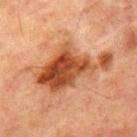Impression:
Recorded during total-body skin imaging; not selected for excision or biopsy.
Image and clinical context:
This is a cross-polarized tile. A male subject approximately 65 years of age. Measured at roughly 9.5 mm in maximum diameter. From the front of the torso. A 15 mm close-up extracted from a 3D total-body photography capture.